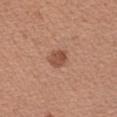Q: Is there a histopathology result?
A: total-body-photography surveillance lesion; no biopsy